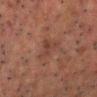Assessment: The lesion was tiled from a total-body skin photograph and was not biopsied. Acquisition and patient details: Approximately 3.5 mm at its widest. This is a cross-polarized tile. A roughly 15 mm field-of-view crop from a total-body skin photograph. A male subject aged approximately 50. On the chest.